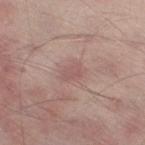The lesion was photographed on a routine skin check and not biopsied; there is no pathology result. A male patient, in their mid- to late 60s. The total-body-photography lesion software estimated an average lesion color of about L≈55 a*≈20 b*≈21 (CIELAB) and a lesion–skin lightness drop of about 7. The analysis additionally found border irregularity of about 2.5 on a 0–10 scale and a peripheral color-asymmetry measure near 0.5. On the right thigh. Captured under white-light illumination. The recorded lesion diameter is about 3 mm. A 15 mm close-up tile from a total-body photography series done for melanoma screening.